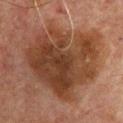The lesion was tiled from a total-body skin photograph and was not biopsied. Located on the upper back. The subject is a male aged approximately 65. Captured under cross-polarized illumination. A lesion tile, about 15 mm wide, cut from a 3D total-body photograph. An algorithmic analysis of the crop reported a footprint of about 50 mm² and two-axis asymmetry of about 0.3. It also reported an average lesion color of about L≈31 a*≈18 b*≈25 (CIELAB), a lesion–skin lightness drop of about 9, and a lesion-to-skin contrast of about 9 (normalized; higher = more distinct). And it measured a border-irregularity index near 6/10 and peripheral color asymmetry of about 1.5. It also reported a classifier nevus-likeness of about 5/100. The lesion's longest dimension is about 9 mm.